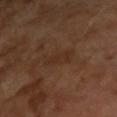The lesion was photographed on a routine skin check and not biopsied; there is no pathology result.
A roughly 15 mm field-of-view crop from a total-body skin photograph.
A male subject about 65 years old.
Measured at roughly 3 mm in maximum diameter.
Captured under cross-polarized illumination.